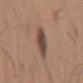Impression:
Recorded during total-body skin imaging; not selected for excision or biopsy.